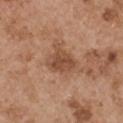{
  "biopsy_status": "not biopsied; imaged during a skin examination",
  "patient": {
    "sex": "male",
    "age_approx": 55
  },
  "automated_metrics": {
    "vs_skin_darker_L": 10.0,
    "vs_skin_contrast_norm": 7.0,
    "border_irregularity_0_10": 3.0,
    "color_variation_0_10": 3.0,
    "peripheral_color_asymmetry": 1.0,
    "nevus_likeness_0_100": 5,
    "lesion_detection_confidence_0_100": 100
  },
  "lighting": "white-light",
  "site": "left upper arm",
  "lesion_size": {
    "long_diameter_mm_approx": 4.0
  },
  "image": {
    "source": "total-body photography crop",
    "field_of_view_mm": 15
  }
}